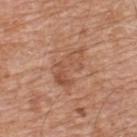Impression: This lesion was catalogued during total-body skin photography and was not selected for biopsy. Image and clinical context: An algorithmic analysis of the crop reported a footprint of about 11 mm², an eccentricity of roughly 0.8, and two-axis asymmetry of about 0.35. About 5.5 mm across. A male subject in their 80s. This is a white-light tile. Cropped from a whole-body photographic skin survey; the tile spans about 15 mm. From the back.